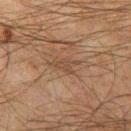Recorded during total-body skin imaging; not selected for excision or biopsy. A male subject, aged 48 to 52. Located on the left thigh. The lesion-visualizer software estimated an outline eccentricity of about 0.8 (0 = round, 1 = elongated). The analysis additionally found a lesion color around L≈38 a*≈14 b*≈25 in CIELAB and a normalized border contrast of about 5. It also reported internal color variation of about 1.5 on a 0–10 scale and a peripheral color-asymmetry measure near 0.5. The analysis additionally found an automated nevus-likeness rating near 0 out of 100 and lesion-presence confidence of about 65/100. The recorded lesion diameter is about 3.5 mm. A 15 mm close-up extracted from a 3D total-body photography capture. Captured under cross-polarized illumination.